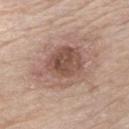Part of a total-body skin-imaging series; this lesion was reviewed on a skin check and was not flagged for biopsy. The tile uses white-light illumination. A male subject about 65 years old. The lesion's longest dimension is about 6 mm. A close-up tile cropped from a whole-body skin photograph, about 15 mm across. The lesion is on the chest. The lesion-visualizer software estimated a classifier nevus-likeness of about 15/100.